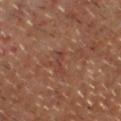The total-body-photography lesion software estimated a lesion area of about 2.5 mm², a shape eccentricity near 0.95, and a symmetry-axis asymmetry near 0.55. The software also gave a border-irregularity rating of about 7/10, a color-variation rating of about 0/10, and radial color variation of about 0. And it measured an automated nevus-likeness rating near 0 out of 100 and a detector confidence of about 90 out of 100 that the crop contains a lesion. The lesion's longest dimension is about 3 mm. Imaged with cross-polarized lighting. A male patient, aged 58 to 62. The lesion is on the head or neck. Cropped from a total-body skin-imaging series; the visible field is about 15 mm.